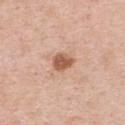Recorded during total-body skin imaging; not selected for excision or biopsy. On the right upper arm. A lesion tile, about 15 mm wide, cut from a 3D total-body photograph. Automated image analysis of the tile measured a lesion area of about 5 mm² and a shape eccentricity near 0.65. The software also gave a border-irregularity index near 2/10, internal color variation of about 2 on a 0–10 scale, and radial color variation of about 0.5. A male patient in their mid- to late 50s.